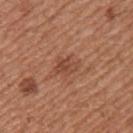Imaged during a routine full-body skin examination; the lesion was not biopsied and no histopathology is available.